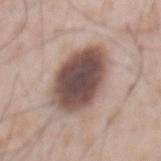The lesion was photographed on a routine skin check and not biopsied; there is no pathology result. On the back. Approximately 7.5 mm at its widest. Cropped from a total-body skin-imaging series; the visible field is about 15 mm. A male patient, in their mid- to late 50s. This is a white-light tile.